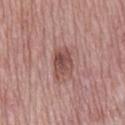Recorded during total-body skin imaging; not selected for excision or biopsy.
A lesion tile, about 15 mm wide, cut from a 3D total-body photograph.
The lesion is on the mid back.
A male subject aged around 75.